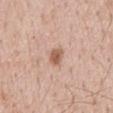The total-body-photography lesion software estimated an area of roughly 3.5 mm², an eccentricity of roughly 0.85, and a symmetry-axis asymmetry near 0.2. The software also gave a mean CIELAB color near L≈58 a*≈21 b*≈30 and a normalized border contrast of about 8.
The patient is a male in their 50s.
Located on the mid back.
Cropped from a whole-body photographic skin survey; the tile spans about 15 mm.